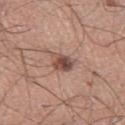workup: catalogued during a skin exam; not biopsied
diameter: ~3 mm (longest diameter)
image-analysis metrics: a mean CIELAB color near L≈49 a*≈20 b*≈25, about 13 CIELAB-L* units darker than the surrounding skin, and a normalized border contrast of about 9; an automated nevus-likeness rating near 90 out of 100 and a lesion-detection confidence of about 100/100
patient: male, aged 38 to 42
location: the left thigh
lighting: white-light illumination
image source: ~15 mm crop, total-body skin-cancer survey